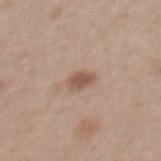Clinical impression:
This lesion was catalogued during total-body skin photography and was not selected for biopsy.
Acquisition and patient details:
This is a white-light tile. A female patient aged around 40. The lesion is on the mid back. The total-body-photography lesion software estimated a shape eccentricity near 0.75 and a symmetry-axis asymmetry near 0.25. And it measured a lesion color around L≈54 a*≈18 b*≈27 in CIELAB, about 11 CIELAB-L* units darker than the surrounding skin, and a normalized border contrast of about 7.5. Approximately 3 mm at its widest. A 15 mm close-up extracted from a 3D total-body photography capture.